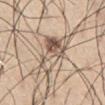<case>
<biopsy_status>not biopsied; imaged during a skin examination</biopsy_status>
<automated_metrics>
  <area_mm2_approx>16.0</area_mm2_approx>
  <eccentricity>0.95</eccentricity>
  <cielab_L>59</cielab_L>
  <cielab_a>13</cielab_a>
  <cielab_b>27</cielab_b>
  <nevus_likeness_0_100>40</nevus_likeness_0_100>
</automated_metrics>
<site>right thigh</site>
<image>
  <source>total-body photography crop</source>
  <field_of_view_mm>15</field_of_view_mm>
</image>
<lesion_size>
  <long_diameter_mm_approx>8.0</long_diameter_mm_approx>
</lesion_size>
<patient>
  <sex>male</sex>
  <age_approx>55</age_approx>
</patient>
</case>